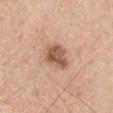Captured during whole-body skin photography for melanoma surveillance; the lesion was not biopsied. Cropped from a total-body skin-imaging series; the visible field is about 15 mm. A male subject in their mid-50s. Located on the arm.